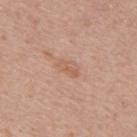Q: Is there a histopathology result?
A: no biopsy performed (imaged during a skin exam)
Q: Who is the patient?
A: male, in their 60s
Q: Where on the body is the lesion?
A: the mid back
Q: How was this image acquired?
A: ~15 mm crop, total-body skin-cancer survey
Q: What did automated image analysis measure?
A: a mean CIELAB color near L≈59 a*≈21 b*≈31 and roughly 7 lightness units darker than nearby skin; a peripheral color-asymmetry measure near 1
Q: How large is the lesion?
A: ~3 mm (longest diameter)